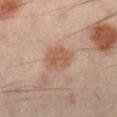A male subject, aged 38–42.
A roughly 15 mm field-of-view crop from a total-body skin photograph.
From the right lower leg.